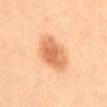{"biopsy_status": "not biopsied; imaged during a skin examination", "patient": {"sex": "female", "age_approx": 35}, "image": {"source": "total-body photography crop", "field_of_view_mm": 15}, "site": "abdomen"}